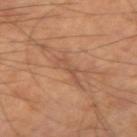{
  "biopsy_status": "not biopsied; imaged during a skin examination",
  "site": "right upper arm",
  "image": {
    "source": "total-body photography crop",
    "field_of_view_mm": 15
  },
  "patient": {
    "sex": "male",
    "age_approx": 60
  }
}